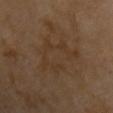No biopsy was performed on this lesion — it was imaged during a full skin examination and was not determined to be concerning.
From the front of the torso.
A close-up tile cropped from a whole-body skin photograph, about 15 mm across.
A male patient, in their mid- to late 50s.